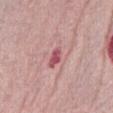{
  "biopsy_status": "not biopsied; imaged during a skin examination",
  "site": "abdomen",
  "lighting": "white-light",
  "patient": {
    "sex": "female",
    "age_approx": 65
  },
  "image": {
    "source": "total-body photography crop",
    "field_of_view_mm": 15
  }
}